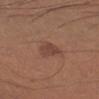* follow-up: total-body-photography surveillance lesion; no biopsy
* image: ~15 mm tile from a whole-body skin photo
* diameter: ≈2.5 mm
* automated lesion analysis: a lesion color around L≈43 a*≈21 b*≈25 in CIELAB; a detector confidence of about 100 out of 100 that the crop contains a lesion
* subject: male, aged 33 to 37
* anatomic site: the left upper arm
* tile lighting: white-light illumination